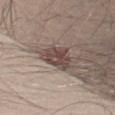Assessment: Part of a total-body skin-imaging series; this lesion was reviewed on a skin check and was not flagged for biopsy. Background: A region of skin cropped from a whole-body photographic capture, roughly 15 mm wide. On the front of the torso. A male subject approximately 40 years of age. Captured under white-light illumination.